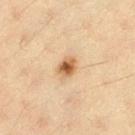notes=catalogued during a skin exam; not biopsied
TBP lesion metrics=a border-irregularity index near 2/10, internal color variation of about 4 on a 0–10 scale, and a peripheral color-asymmetry measure near 1
site=the left thigh
image=15 mm crop, total-body photography
patient=female, about 40 years old
lesion size=about 2.5 mm